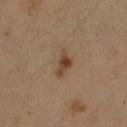Recorded during total-body skin imaging; not selected for excision or biopsy.
Approximately 3.5 mm at its widest.
A lesion tile, about 15 mm wide, cut from a 3D total-body photograph.
The patient is a male approximately 50 years of age.
On the left arm.
This is a cross-polarized tile.
Automated image analysis of the tile measured a footprint of about 5 mm², an outline eccentricity of about 0.85 (0 = round, 1 = elongated), and a symmetry-axis asymmetry near 0.35. The analysis additionally found border irregularity of about 3.5 on a 0–10 scale, internal color variation of about 4.5 on a 0–10 scale, and peripheral color asymmetry of about 1.5. The software also gave a detector confidence of about 100 out of 100 that the crop contains a lesion.